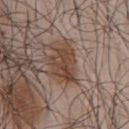Impression: Part of a total-body skin-imaging series; this lesion was reviewed on a skin check and was not flagged for biopsy. Image and clinical context: Cropped from a total-body skin-imaging series; the visible field is about 15 mm. The lesion is on the chest. The subject is a male aged approximately 45.